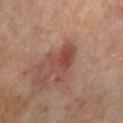This lesion was catalogued during total-body skin photography and was not selected for biopsy. Longest diameter approximately 5 mm. The lesion is on the leg. A male patient, approximately 70 years of age. Imaged with cross-polarized lighting. A lesion tile, about 15 mm wide, cut from a 3D total-body photograph.